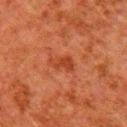From the right upper arm.
Captured under cross-polarized illumination.
Longest diameter approximately 3 mm.
The patient is a male aged 78–82.
A 15 mm crop from a total-body photograph taken for skin-cancer surveillance.